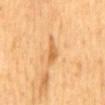This lesion was catalogued during total-body skin photography and was not selected for biopsy. Automated tile analysis of the lesion measured an eccentricity of roughly 0.9. The analysis additionally found a lesion color around L≈66 a*≈24 b*≈46 in CIELAB and a lesion–skin lightness drop of about 10. The analysis additionally found border irregularity of about 4.5 on a 0–10 scale, a within-lesion color-variation index near 2.5/10, and peripheral color asymmetry of about 1. A 15 mm close-up tile from a total-body photography series done for melanoma screening. A male subject in their mid- to late 60s. Captured under cross-polarized illumination. On the mid back. The recorded lesion diameter is about 3.5 mm.